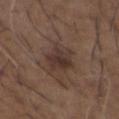Impression: The lesion was tiled from a total-body skin photograph and was not biopsied.